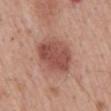This lesion was catalogued during total-body skin photography and was not selected for biopsy. A 15 mm close-up tile from a total-body photography series done for melanoma screening. Imaged with white-light lighting. The lesion is located on the mid back. The subject is a male aged approximately 65.